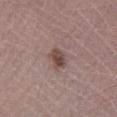- body site: the left lower leg
- acquisition: ~15 mm tile from a whole-body skin photo
- lesion diameter: ~3 mm (longest diameter)
- tile lighting: white-light
- patient: female, aged 48 to 52